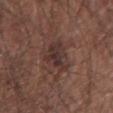Captured during whole-body skin photography for melanoma surveillance; the lesion was not biopsied.
Automated image analysis of the tile measured a lesion color around L≈33 a*≈17 b*≈20 in CIELAB and a normalized border contrast of about 7.5. The software also gave a nevus-likeness score of about 5/100.
Cropped from a total-body skin-imaging series; the visible field is about 15 mm.
From the left upper arm.
Measured at roughly 4.5 mm in maximum diameter.
Captured under white-light illumination.
A male patient aged 63 to 67.